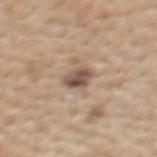A female subject, aged 48–52. A 15 mm close-up tile from a total-body photography series done for melanoma screening. Automated image analysis of the tile measured border irregularity of about 1.5 on a 0–10 scale, a color-variation rating of about 5/10, and a peripheral color-asymmetry measure near 1.5. It also reported an automated nevus-likeness rating near 45 out of 100. The tile uses white-light illumination. From the upper back.